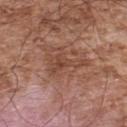No biopsy was performed on this lesion — it was imaged during a full skin examination and was not determined to be concerning. The lesion's longest dimension is about 4.5 mm. Automated image analysis of the tile measured an eccentricity of roughly 0.85 and a symmetry-axis asymmetry near 0.6. From the back. A male patient about 55 years old. A roughly 15 mm field-of-view crop from a total-body skin photograph. This is a white-light tile.